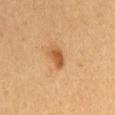Clinical impression: Imaged during a routine full-body skin examination; the lesion was not biopsied and no histopathology is available. Clinical summary: A lesion tile, about 15 mm wide, cut from a 3D total-body photograph. A male patient, aged 43 to 47. Approximately 2.5 mm at its widest. Located on the chest. The tile uses cross-polarized illumination.